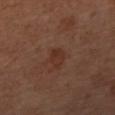notes: no biopsy performed (imaged during a skin exam).